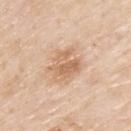Q: Is there a histopathology result?
A: total-body-photography surveillance lesion; no biopsy
Q: What kind of image is this?
A: ~15 mm crop, total-body skin-cancer survey
Q: What did automated image analysis measure?
A: a border-irregularity rating of about 3/10, internal color variation of about 5 on a 0–10 scale, and peripheral color asymmetry of about 2; an automated nevus-likeness rating near 5 out of 100 and a lesion-detection confidence of about 100/100
Q: What are the patient's age and sex?
A: male, aged around 80
Q: Where on the body is the lesion?
A: the upper back
Q: How large is the lesion?
A: about 4.5 mm
Q: How was the tile lit?
A: white-light illumination